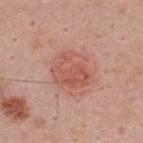The lesion is located on the back.
A 15 mm close-up extracted from a 3D total-body photography capture.
A male subject about 45 years old.
Approximately 4.5 mm at its widest.
Captured under white-light illumination.